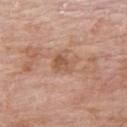Assessment:
The lesion was photographed on a routine skin check and not biopsied; there is no pathology result.
Image and clinical context:
On the upper back. The recorded lesion diameter is about 2.5 mm. Cropped from a total-body skin-imaging series; the visible field is about 15 mm. A female patient, about 70 years old.